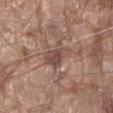Clinical summary:
The tile uses white-light illumination. The lesion-visualizer software estimated a border-irregularity index near 4/10. The analysis additionally found an automated nevus-likeness rating near 0 out of 100 and a detector confidence of about 95 out of 100 that the crop contains a lesion. A male patient approximately 80 years of age. A close-up tile cropped from a whole-body skin photograph, about 15 mm across. The lesion is located on the abdomen.